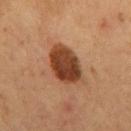Acquisition and patient details:
A 15 mm close-up tile from a total-body photography series done for melanoma screening. From the mid back. Captured under cross-polarized illumination. A male subject approximately 55 years of age. The lesion's longest dimension is about 5.5 mm.